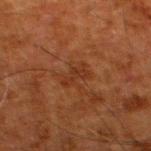  biopsy_status: not biopsied; imaged during a skin examination
  image:
    source: total-body photography crop
    field_of_view_mm: 15
  patient:
    sex: male
    age_approx: 80
  lesion_size:
    long_diameter_mm_approx: 3.0
  lighting: cross-polarized
  site: leg
  automated_metrics:
    cielab_L: 26
    cielab_a: 21
    cielab_b: 28
    vs_skin_darker_L: 5.0
    vs_skin_contrast_norm: 6.0